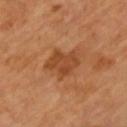{
  "biopsy_status": "not biopsied; imaged during a skin examination",
  "site": "chest",
  "patient": {
    "sex": "male",
    "age_approx": 65
  },
  "lesion_size": {
    "long_diameter_mm_approx": 4.0
  },
  "image": {
    "source": "total-body photography crop",
    "field_of_view_mm": 15
  },
  "automated_metrics": {
    "eccentricity": 0.65,
    "shape_asymmetry": 0.3,
    "cielab_L": 44,
    "cielab_a": 24,
    "cielab_b": 37,
    "vs_skin_darker_L": 9.0,
    "vs_skin_contrast_norm": 7.0,
    "color_variation_0_10": 2.5,
    "peripheral_color_asymmetry": 1.0,
    "lesion_detection_confidence_0_100": 100
  },
  "lighting": "cross-polarized"
}